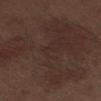<tbp_lesion>
<biopsy_status>not biopsied; imaged during a skin examination</biopsy_status>
<automated_metrics>
  <cielab_L>29</cielab_L>
  <cielab_a>15</cielab_a>
  <cielab_b>19</cielab_b>
  <vs_skin_contrast_norm>6.0</vs_skin_contrast_norm>
  <border_irregularity_0_10>9.0</border_irregularity_0_10>
  <color_variation_0_10>4.0</color_variation_0_10>
  <peripheral_color_asymmetry>1.5</peripheral_color_asymmetry>
  <lesion_detection_confidence_0_100>100</lesion_detection_confidence_0_100>
</automated_metrics>
<patient>
  <sex>male</sex>
  <age_approx>70</age_approx>
</patient>
<lesion_size>
  <long_diameter_mm_approx>13.5</long_diameter_mm_approx>
</lesion_size>
<image>
  <source>total-body photography crop</source>
  <field_of_view_mm>15</field_of_view_mm>
</image>
<lighting>white-light</lighting>
<site>right thigh</site>
</tbp_lesion>